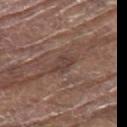notes: imaged on a skin check; not biopsied
lesion diameter: about 2.5 mm
lighting: white-light illumination
automated metrics: an area of roughly 3.5 mm² and a shape eccentricity near 0.75; an automated nevus-likeness rating near 0 out of 100 and a lesion-detection confidence of about 55/100
imaging modality: ~15 mm tile from a whole-body skin photo
location: the upper back
subject: male, about 80 years old